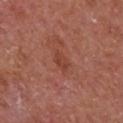biopsy status=total-body-photography surveillance lesion; no biopsy | acquisition=total-body-photography crop, ~15 mm field of view | size=≈2.5 mm | site=the chest | image-analysis metrics=a mean CIELAB color near L≈42 a*≈27 b*≈30, roughly 7 lightness units darker than nearby skin, and a normalized lesion–skin contrast near 6 | subject=male, about 65 years old.